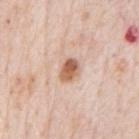{
  "biopsy_status": "not biopsied; imaged during a skin examination",
  "lesion_size": {
    "long_diameter_mm_approx": 3.0
  },
  "site": "chest",
  "lighting": "white-light",
  "patient": {
    "sex": "male",
    "age_approx": 80
  },
  "automated_metrics": {
    "area_mm2_approx": 5.0,
    "eccentricity": 0.7,
    "shape_asymmetry": 0.25,
    "cielab_L": 61,
    "cielab_a": 22,
    "cielab_b": 32,
    "vs_skin_contrast_norm": 10.0,
    "border_irregularity_0_10": 2.5,
    "peripheral_color_asymmetry": 2.0,
    "nevus_likeness_0_100": 95,
    "lesion_detection_confidence_0_100": 100
  },
  "image": {
    "source": "total-body photography crop",
    "field_of_view_mm": 15
  }
}